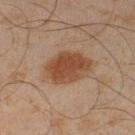Findings:
* follow-up — no biopsy performed (imaged during a skin exam)
* automated lesion analysis — a footprint of about 15 mm²; a mean CIELAB color near L≈36 a*≈18 b*≈26, about 9 CIELAB-L* units darker than the surrounding skin, and a normalized lesion–skin contrast near 9
* lesion diameter — ≈5.5 mm
* anatomic site — the left lower leg
* patient — male, approximately 45 years of age
* image — 15 mm crop, total-body photography
* lighting — cross-polarized